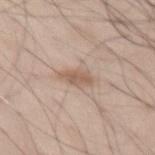Recorded during total-body skin imaging; not selected for excision or biopsy. The total-body-photography lesion software estimated a nevus-likeness score of about 20/100. The lesion is on the left thigh. The patient is a male approximately 45 years of age. A close-up tile cropped from a whole-body skin photograph, about 15 mm across. The recorded lesion diameter is about 3 mm.